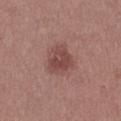automated metrics: a footprint of about 8.5 mm², an outline eccentricity of about 0.6 (0 = round, 1 = elongated), and two-axis asymmetry of about 0.25; an automated nevus-likeness rating near 60 out of 100 and a lesion-detection confidence of about 100/100
patient: female, about 35 years old
imaging modality: total-body-photography crop, ~15 mm field of view
illumination: white-light illumination
diameter: ≈3.5 mm
anatomic site: the front of the torso